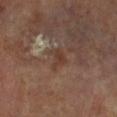Q: Was a biopsy performed?
A: catalogued during a skin exam; not biopsied
Q: What are the patient's age and sex?
A: female, about 75 years old
Q: How was the tile lit?
A: cross-polarized illumination
Q: What kind of image is this?
A: ~15 mm crop, total-body skin-cancer survey
Q: What is the anatomic site?
A: the left lower leg
Q: Lesion size?
A: ~3 mm (longest diameter)